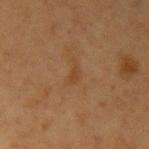The lesion was photographed on a routine skin check and not biopsied; there is no pathology result.
Captured under cross-polarized illumination.
The lesion's longest dimension is about 2.5 mm.
On the arm.
A region of skin cropped from a whole-body photographic capture, roughly 15 mm wide.
The patient is a female aged 38 to 42.